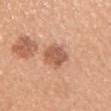workup = no biopsy performed (imaged during a skin exam)
TBP lesion metrics = a classifier nevus-likeness of about 5/100
anatomic site = the left upper arm
patient = female, about 30 years old
lighting = white-light illumination
acquisition = 15 mm crop, total-body photography
lesion diameter = ~3.5 mm (longest diameter)